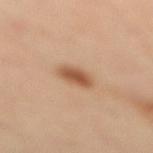{"biopsy_status": "not biopsied; imaged during a skin examination", "patient": {"sex": "female", "age_approx": 40}, "lighting": "cross-polarized", "site": "left leg", "image": {"source": "total-body photography crop", "field_of_view_mm": 15}, "lesion_size": {"long_diameter_mm_approx": 3.0}}